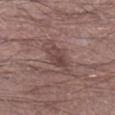location — the left lower leg | tile lighting — white-light illumination | acquisition — ~15 mm crop, total-body skin-cancer survey | subject — male, roughly 45 years of age | image-analysis metrics — a lesion area of about 5.5 mm², an outline eccentricity of about 0.85 (0 = round, 1 = elongated), and two-axis asymmetry of about 0.25; border irregularity of about 2.5 on a 0–10 scale, a color-variation rating of about 4.5/10, and peripheral color asymmetry of about 2.